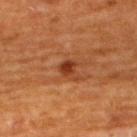Impression:
Recorded during total-body skin imaging; not selected for excision or biopsy.
Background:
The lesion is on the back. Captured under cross-polarized illumination. The total-body-photography lesion software estimated a footprint of about 3.5 mm², an eccentricity of roughly 0.4, and a shape-asymmetry score of about 0.35 (0 = symmetric). And it measured an automated nevus-likeness rating near 65 out of 100 and a detector confidence of about 100 out of 100 that the crop contains a lesion. This image is a 15 mm lesion crop taken from a total-body photograph. A male subject, in their 60s.